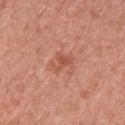Image and clinical context:
An algorithmic analysis of the crop reported a lesion color around L≈54 a*≈28 b*≈32 in CIELAB, about 8 CIELAB-L* units darker than the surrounding skin, and a normalized lesion–skin contrast near 6. A 15 mm close-up extracted from a 3D total-body photography capture. The lesion is on the arm. Imaged with white-light lighting. A female subject, approximately 40 years of age. About 2.5 mm across.